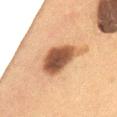Captured during whole-body skin photography for melanoma surveillance; the lesion was not biopsied. Located on the abdomen. Captured under cross-polarized illumination. A female patient aged approximately 50. Approximately 6 mm at its widest. An algorithmic analysis of the crop reported border irregularity of about 3 on a 0–10 scale, a within-lesion color-variation index near 6/10, and radial color variation of about 1.5. A 15 mm close-up tile from a total-body photography series done for melanoma screening.